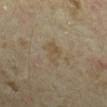biopsy status — no biopsy performed (imaged during a skin exam)
site — the left forearm
patient — female, aged 33–37
lesion diameter — about 3.5 mm
imaging modality — 15 mm crop, total-body photography